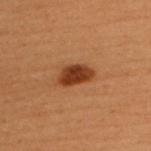<lesion>
<biopsy_status>not biopsied; imaged during a skin examination</biopsy_status>
<patient>
  <sex>female</sex>
  <age_approx>50</age_approx>
</patient>
<site>back</site>
<lesion_size>
  <long_diameter_mm_approx>4.0</long_diameter_mm_approx>
</lesion_size>
<image>
  <source>total-body photography crop</source>
  <field_of_view_mm>15</field_of_view_mm>
</image>
<lighting>cross-polarized</lighting>
</lesion>